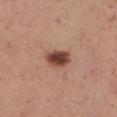{"biopsy_status": "not biopsied; imaged during a skin examination", "lighting": "white-light", "image": {"source": "total-body photography crop", "field_of_view_mm": 15}, "patient": {"sex": "female", "age_approx": 30}, "site": "left thigh", "lesion_size": {"long_diameter_mm_approx": 3.0}, "automated_metrics": {"cielab_L": 45, "cielab_a": 22, "cielab_b": 27, "vs_skin_darker_L": 16.0, "vs_skin_contrast_norm": 11.5, "nevus_likeness_0_100": 95, "lesion_detection_confidence_0_100": 100}}